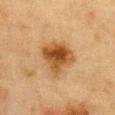Recorded during total-body skin imaging; not selected for excision or biopsy. The lesion is located on the chest. Longest diameter approximately 4.5 mm. The subject is a female approximately 55 years of age. Captured under cross-polarized illumination. A region of skin cropped from a whole-body photographic capture, roughly 15 mm wide. The lesion-visualizer software estimated a footprint of about 15 mm², an outline eccentricity of about 0.45 (0 = round, 1 = elongated), and a shape-asymmetry score of about 0.3 (0 = symmetric). The software also gave a mean CIELAB color near L≈43 a*≈19 b*≈35, roughly 12 lightness units darker than nearby skin, and a normalized border contrast of about 10. The software also gave a color-variation rating of about 7.5/10 and radial color variation of about 2.5. The analysis additionally found a nevus-likeness score of about 95/100 and lesion-presence confidence of about 100/100.